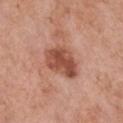Notes:
• biopsy status · no biopsy performed (imaged during a skin exam)
• diameter · ~5 mm (longest diameter)
• tile lighting · white-light
• patient · female, aged around 60
• image · 15 mm crop, total-body photography
• site · the chest